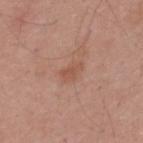| key | value |
|---|---|
| notes | catalogued during a skin exam; not biopsied |
| body site | the upper back |
| diameter | ~3 mm (longest diameter) |
| acquisition | ~15 mm crop, total-body skin-cancer survey |
| subject | male, approximately 40 years of age |